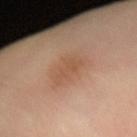This lesion was catalogued during total-body skin photography and was not selected for biopsy. Captured under cross-polarized illumination. A male subject, aged approximately 50. The total-body-photography lesion software estimated a lesion area of about 5.5 mm², a shape eccentricity near 0.8, and two-axis asymmetry of about 0.4. It also reported border irregularity of about 4.5 on a 0–10 scale and a within-lesion color-variation index near 1.5/10. The software also gave an automated nevus-likeness rating near 25 out of 100 and a lesion-detection confidence of about 100/100. The lesion is located on the left forearm. Longest diameter approximately 3.5 mm. Cropped from a whole-body photographic skin survey; the tile spans about 15 mm.